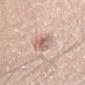Case summary:
- notes — catalogued during a skin exam; not biopsied
- anatomic site — the right thigh
- image-analysis metrics — border irregularity of about 3.5 on a 0–10 scale, internal color variation of about 6.5 on a 0–10 scale, and radial color variation of about 2.5
- size — ≈4 mm
- patient — male, aged around 40
- imaging modality — ~15 mm crop, total-body skin-cancer survey